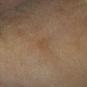A female patient, roughly 60 years of age. Automated tile analysis of the lesion measured an area of roughly 4 mm² and a shape-asymmetry score of about 0.3 (0 = symmetric). The software also gave a border-irregularity rating of about 3/10, internal color variation of about 1 on a 0–10 scale, and radial color variation of about 0.5. The analysis additionally found a nevus-likeness score of about 0/100 and lesion-presence confidence of about 100/100. The lesion is on the left forearm. Longest diameter approximately 3.5 mm. A 15 mm close-up extracted from a 3D total-body photography capture. Captured under cross-polarized illumination.